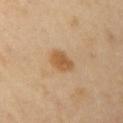{"automated_metrics": {"cielab_L": 57, "cielab_a": 19, "cielab_b": 38, "vs_skin_darker_L": 10.0, "vs_skin_contrast_norm": 7.5, "nevus_likeness_0_100": 80, "lesion_detection_confidence_0_100": 100}, "patient": {"sex": "female", "age_approx": 45}, "site": "left upper arm", "image": {"source": "total-body photography crop", "field_of_view_mm": 15}, "lesion_size": {"long_diameter_mm_approx": 3.0}}